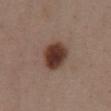<record>
  <lesion_size>
    <long_diameter_mm_approx>4.0</long_diameter_mm_approx>
  </lesion_size>
  <image>
    <source>total-body photography crop</source>
    <field_of_view_mm>15</field_of_view_mm>
  </image>
  <patient>
    <sex>female</sex>
    <age_approx>55</age_approx>
  </patient>
  <lighting>white-light</lighting>
  <automated_metrics>
    <border_irregularity_0_10>1.5</border_irregularity_0_10>
    <color_variation_0_10>5.0</color_variation_0_10>
    <nevus_likeness_0_100>100</nevus_likeness_0_100>
    <lesion_detection_confidence_0_100>100</lesion_detection_confidence_0_100>
  </automated_metrics>
  <site>abdomen</site>
</record>